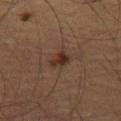No biopsy was performed on this lesion — it was imaged during a full skin examination and was not determined to be concerning. This is a cross-polarized tile. From the left thigh. The patient is a male approximately 65 years of age. Cropped from a whole-body photographic skin survey; the tile spans about 15 mm. Automated image analysis of the tile measured a mean CIELAB color near L≈25 a*≈14 b*≈21, a lesion–skin lightness drop of about 7, and a normalized border contrast of about 8.5. The software also gave a border-irregularity rating of about 3/10, internal color variation of about 3 on a 0–10 scale, and peripheral color asymmetry of about 1. It also reported a nevus-likeness score of about 80/100 and lesion-presence confidence of about 100/100. The recorded lesion diameter is about 2.5 mm.